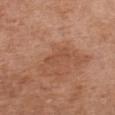Assessment:
The lesion was tiled from a total-body skin photograph and was not biopsied.
Context:
The recorded lesion diameter is about 3.5 mm. A 15 mm close-up extracted from a 3D total-body photography capture. Automated tile analysis of the lesion measured about 6 CIELAB-L* units darker than the surrounding skin. The software also gave a lesion-detection confidence of about 100/100. Located on the chest. The patient is a female aged 63 to 67. Captured under white-light illumination.